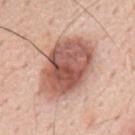{
  "biopsy_status": "not biopsied; imaged during a skin examination",
  "image": {
    "source": "total-body photography crop",
    "field_of_view_mm": 15
  },
  "site": "mid back",
  "automated_metrics": {
    "cielab_L": 57,
    "cielab_a": 23,
    "cielab_b": 27,
    "vs_skin_darker_L": 16.0,
    "vs_skin_contrast_norm": 10.0,
    "border_irregularity_0_10": 1.5,
    "color_variation_0_10": 7.0,
    "peripheral_color_asymmetry": 2.5,
    "nevus_likeness_0_100": 85
  },
  "patient": {
    "sex": "male",
    "age_approx": 60
  },
  "lighting": "white-light"
}